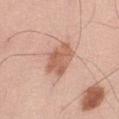Case summary:
- notes: total-body-photography surveillance lesion; no biopsy
- lighting: white-light illumination
- lesion diameter: ≈4 mm
- site: the abdomen
- imaging modality: ~15 mm tile from a whole-body skin photo
- patient: male, aged 68–72